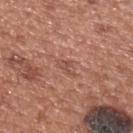Findings:
- notes: no biopsy performed (imaged during a skin exam)
- location: the upper back
- illumination: white-light illumination
- image source: total-body-photography crop, ~15 mm field of view
- lesion diameter: about 2.5 mm
- patient: male, aged approximately 55